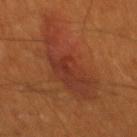| feature | finding |
|---|---|
| illumination | cross-polarized illumination |
| patient | male, aged approximately 60 |
| acquisition | ~15 mm crop, total-body skin-cancer survey |
| location | the mid back |
| diameter | ~10 mm (longest diameter) |